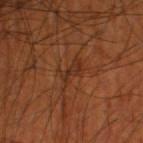Impression:
Imaged during a routine full-body skin examination; the lesion was not biopsied and no histopathology is available.
Context:
The lesion's longest dimension is about 3.5 mm. Captured under cross-polarized illumination. Automated tile analysis of the lesion measured a footprint of about 5 mm² and two-axis asymmetry of about 0.5. It also reported a border-irregularity index near 7/10, a color-variation rating of about 1.5/10, and a peripheral color-asymmetry measure near 0.5. Cropped from a whole-body photographic skin survey; the tile spans about 15 mm. A male patient aged around 55. From the right thigh.